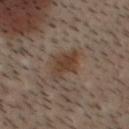Clinical impression:
No biopsy was performed on this lesion — it was imaged during a full skin examination and was not determined to be concerning.
Background:
The lesion-visualizer software estimated border irregularity of about 3.5 on a 0–10 scale and a color-variation rating of about 2.5/10. It also reported a classifier nevus-likeness of about 60/100 and a lesion-detection confidence of about 100/100. Approximately 4 mm at its widest. The lesion is on the head or neck. The patient is a male aged 28 to 32. Cropped from a total-body skin-imaging series; the visible field is about 15 mm.